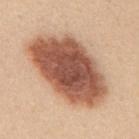Part of a total-body skin-imaging series; this lesion was reviewed on a skin check and was not flagged for biopsy.
An algorithmic analysis of the crop reported a lesion area of about 47 mm², a shape eccentricity near 0.85, and two-axis asymmetry of about 0.15. The software also gave internal color variation of about 6.5 on a 0–10 scale.
The subject is a female aged around 45.
The lesion is located on the mid back.
The recorded lesion diameter is about 10 mm.
This is a white-light tile.
A close-up tile cropped from a whole-body skin photograph, about 15 mm across.